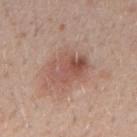Case summary:
- notes — no biopsy performed (imaged during a skin exam)
- image — ~15 mm tile from a whole-body skin photo
- patient — male, in their 30s
- location — the right upper arm